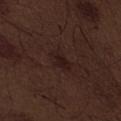The lesion was tiled from a total-body skin photograph and was not biopsied.
The patient is a male roughly 70 years of age.
The recorded lesion diameter is about 3.5 mm.
Automated image analysis of the tile measured a lesion area of about 5.5 mm², a shape eccentricity near 0.85, and two-axis asymmetry of about 0.3. The software also gave peripheral color asymmetry of about 1. The software also gave a nevus-likeness score of about 0/100 and a lesion-detection confidence of about 100/100.
Located on the front of the torso.
A 15 mm close-up extracted from a 3D total-body photography capture.
Imaged with white-light lighting.